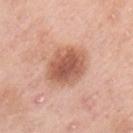follow-up: imaged on a skin check; not biopsied | acquisition: 15 mm crop, total-body photography | subject: female, aged 48 to 52 | illumination: white-light illumination | image-analysis metrics: a nevus-likeness score of about 85/100 and a detector confidence of about 100 out of 100 that the crop contains a lesion | diameter: about 5 mm | anatomic site: the left upper arm.